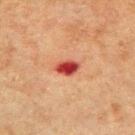Assessment:
Imaged during a routine full-body skin examination; the lesion was not biopsied and no histopathology is available.
Clinical summary:
Imaged with cross-polarized lighting. This image is a 15 mm lesion crop taken from a total-body photograph. The recorded lesion diameter is about 3 mm. The lesion-visualizer software estimated a footprint of about 4.5 mm², an eccentricity of roughly 0.75, and a shape-asymmetry score of about 0.25 (0 = symmetric). It also reported a nevus-likeness score of about 0/100 and lesion-presence confidence of about 100/100. Located on the right upper arm. A male subject, aged 63–67.